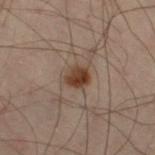Assessment: Recorded during total-body skin imaging; not selected for excision or biopsy. Context: Automated image analysis of the tile measured an area of roughly 5 mm² and an eccentricity of roughly 0.4. It also reported an average lesion color of about L≈31 a*≈15 b*≈23 (CIELAB) and about 10 CIELAB-L* units darker than the surrounding skin. It also reported a border-irregularity rating of about 1.5/10, a within-lesion color-variation index near 4/10, and a peripheral color-asymmetry measure near 1.5. The analysis additionally found an automated nevus-likeness rating near 100 out of 100 and a detector confidence of about 100 out of 100 that the crop contains a lesion. Captured under cross-polarized illumination. On the left leg. A male patient aged approximately 50. This image is a 15 mm lesion crop taken from a total-body photograph.